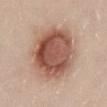Part of a total-body skin-imaging series; this lesion was reviewed on a skin check and was not flagged for biopsy.
About 7.5 mm across.
A female subject in their 30s.
Imaged with white-light lighting.
An algorithmic analysis of the crop reported a mean CIELAB color near L≈54 a*≈22 b*≈28 and roughly 15 lightness units darker than nearby skin. The analysis additionally found an automated nevus-likeness rating near 100 out of 100 and a detector confidence of about 100 out of 100 that the crop contains a lesion.
The lesion is located on the front of the torso.
Cropped from a total-body skin-imaging series; the visible field is about 15 mm.